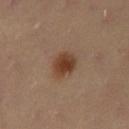<case>
<biopsy_status>not biopsied; imaged during a skin examination</biopsy_status>
<patient>
  <sex>female</sex>
  <age_approx>40</age_approx>
</patient>
<lesion_size>
  <long_diameter_mm_approx>3.5</long_diameter_mm_approx>
</lesion_size>
<site>left thigh</site>
<lighting>cross-polarized</lighting>
<image>
  <source>total-body photography crop</source>
  <field_of_view_mm>15</field_of_view_mm>
</image>
</case>